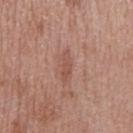{"biopsy_status": "not biopsied; imaged during a skin examination", "lighting": "white-light", "patient": {"sex": "male", "age_approx": 65}, "image": {"source": "total-body photography crop", "field_of_view_mm": 15}, "site": "mid back", "lesion_size": {"long_diameter_mm_approx": 3.5}}